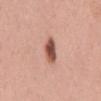Clinical impression:
The lesion was tiled from a total-body skin photograph and was not biopsied.